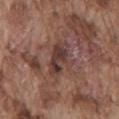The lesion was tiled from a total-body skin photograph and was not biopsied.
A region of skin cropped from a whole-body photographic capture, roughly 15 mm wide.
Captured under white-light illumination.
From the back.
A male subject about 75 years old.